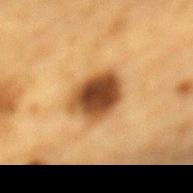Acquisition and patient details:
The lesion is on the mid back. The recorded lesion diameter is about 5 mm. Automated tile analysis of the lesion measured a lesion area of about 14 mm², an eccentricity of roughly 0.65, and a shape-asymmetry score of about 0.2 (0 = symmetric). The software also gave border irregularity of about 2 on a 0–10 scale, a color-variation rating of about 8/10, and radial color variation of about 2.5. A male patient, in their mid-80s or older. A region of skin cropped from a whole-body photographic capture, roughly 15 mm wide.